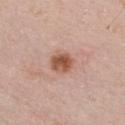Clinical impression:
Imaged during a routine full-body skin examination; the lesion was not biopsied and no histopathology is available.
Context:
Measured at roughly 3 mm in maximum diameter. Automated tile analysis of the lesion measured an area of roughly 6.5 mm², an eccentricity of roughly 0.45, and a symmetry-axis asymmetry near 0.15. It also reported an average lesion color of about L≈55 a*≈23 b*≈30 (CIELAB), about 13 CIELAB-L* units darker than the surrounding skin, and a normalized lesion–skin contrast near 9.5. It also reported a classifier nevus-likeness of about 95/100 and a lesion-detection confidence of about 100/100. The lesion is located on the front of the torso. A region of skin cropped from a whole-body photographic capture, roughly 15 mm wide. The patient is a male about 60 years old.